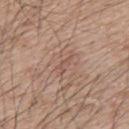Q: Was a biopsy performed?
A: catalogued during a skin exam; not biopsied
Q: What are the patient's age and sex?
A: male, aged 63 to 67
Q: Lesion location?
A: the upper back
Q: How was this image acquired?
A: ~15 mm tile from a whole-body skin photo